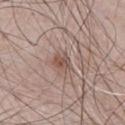Q: Was a biopsy performed?
A: no biopsy performed (imaged during a skin exam)
Q: How large is the lesion?
A: about 2.5 mm
Q: What are the patient's age and sex?
A: male, in their mid- to late 60s
Q: What is the anatomic site?
A: the abdomen
Q: Illumination type?
A: white-light
Q: What is the imaging modality?
A: 15 mm crop, total-body photography
Q: What did automated image analysis measure?
A: a lesion color around L≈52 a*≈17 b*≈24 in CIELAB and roughly 9 lightness units darker than nearby skin; a color-variation rating of about 3.5/10 and peripheral color asymmetry of about 1; an automated nevus-likeness rating near 55 out of 100